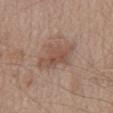• lighting · white-light
• TBP lesion metrics · a lesion area of about 12 mm², an eccentricity of roughly 0.7, and two-axis asymmetry of about 0.25; an average lesion color of about L≈51 a*≈18 b*≈26 (CIELAB) and a normalized border contrast of about 6; border irregularity of about 3.5 on a 0–10 scale, internal color variation of about 3 on a 0–10 scale, and peripheral color asymmetry of about 1; a lesion-detection confidence of about 100/100
• patient · male, aged 68 to 72
• lesion size · ~4 mm (longest diameter)
• body site · the front of the torso
• image · ~15 mm crop, total-body skin-cancer survey